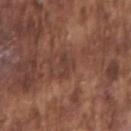Notes:
- follow-up — imaged on a skin check; not biopsied
- tile lighting — white-light illumination
- image — ~15 mm tile from a whole-body skin photo
- anatomic site — the right upper arm
- automated lesion analysis — two-axis asymmetry of about 0.5; a lesion color around L≈39 a*≈20 b*≈24 in CIELAB, a lesion–skin lightness drop of about 7, and a normalized lesion–skin contrast near 6.5; a border-irregularity index near 5.5/10, internal color variation of about 1 on a 0–10 scale, and a peripheral color-asymmetry measure near 0; a detector confidence of about 95 out of 100 that the crop contains a lesion
- lesion size — about 2.5 mm
- patient — male, aged 73–77